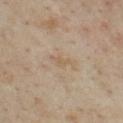Q: Where on the body is the lesion?
A: the chest
Q: What are the patient's age and sex?
A: male, approximately 55 years of age
Q: What kind of image is this?
A: 15 mm crop, total-body photography